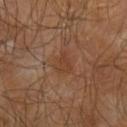<tbp_lesion>
  <biopsy_status>not biopsied; imaged during a skin examination</biopsy_status>
  <site>left upper arm</site>
  <image>
    <source>total-body photography crop</source>
    <field_of_view_mm>15</field_of_view_mm>
  </image>
  <patient>
    <sex>male</sex>
    <age_approx>65</age_approx>
  </patient>
  <lesion_size>
    <long_diameter_mm_approx>3.0</long_diameter_mm_approx>
  </lesion_size>
</tbp_lesion>